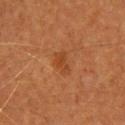Captured during whole-body skin photography for melanoma surveillance; the lesion was not biopsied. The lesion is located on the head or neck. A roughly 15 mm field-of-view crop from a total-body skin photograph. The patient is a female aged approximately 50.